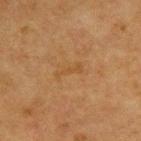Q: Is there a histopathology result?
A: total-body-photography surveillance lesion; no biopsy
Q: How was the tile lit?
A: cross-polarized
Q: Who is the patient?
A: male, in their mid- to late 70s
Q: What kind of image is this?
A: ~15 mm crop, total-body skin-cancer survey
Q: What is the anatomic site?
A: the upper back
Q: Lesion size?
A: about 3 mm